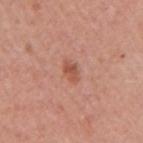The lesion was tiled from a total-body skin photograph and was not biopsied.
The subject is a female aged approximately 50.
A close-up tile cropped from a whole-body skin photograph, about 15 mm across.
Located on the right upper arm.
The lesion's longest dimension is about 2.5 mm.
The tile uses white-light illumination.